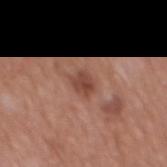Clinical impression:
This lesion was catalogued during total-body skin photography and was not selected for biopsy.
Acquisition and patient details:
A 15 mm close-up extracted from a 3D total-body photography capture. The patient is a male aged approximately 45. On the chest.